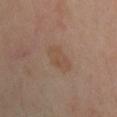This lesion was catalogued during total-body skin photography and was not selected for biopsy. The patient is a female aged around 50. Located on the leg. An algorithmic analysis of the crop reported a classifier nevus-likeness of about 10/100 and lesion-presence confidence of about 100/100. A lesion tile, about 15 mm wide, cut from a 3D total-body photograph.